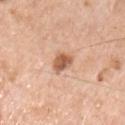  biopsy_status: not biopsied; imaged during a skin examination
  lesion_size:
    long_diameter_mm_approx: 3.0
  automated_metrics:
    nevus_likeness_0_100: 90
  patient:
    sex: male
    age_approx: 70
  site: front of the torso
  image:
    source: total-body photography crop
    field_of_view_mm: 15
  lighting: white-light